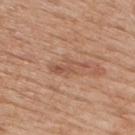Notes:
- follow-up · catalogued during a skin exam; not biopsied
- location · the back
- size · about 4.5 mm
- patient · male, aged 58 to 62
- tile lighting · white-light
- image source · ~15 mm crop, total-body skin-cancer survey